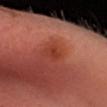- notes: no biopsy performed (imaged during a skin exam)
- automated metrics: a footprint of about 3.5 mm² and two-axis asymmetry of about 0.25; a classifier nevus-likeness of about 0/100
- patient: male, aged around 40
- anatomic site: the head or neck
- image source: ~15 mm tile from a whole-body skin photo
- lesion diameter: about 2.5 mm
- lighting: cross-polarized